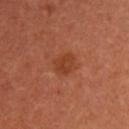Assessment:
Captured during whole-body skin photography for melanoma surveillance; the lesion was not biopsied.
Acquisition and patient details:
Captured under cross-polarized illumination. A female patient in their 50s. The lesion-visualizer software estimated an area of roughly 4.5 mm², a shape eccentricity near 0.7, and a symmetry-axis asymmetry near 0.25. Approximately 2.5 mm at its widest. A 15 mm close-up tile from a total-body photography series done for melanoma screening.